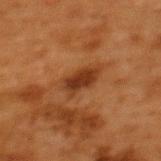On the upper back. The tile uses cross-polarized illumination. A roughly 15 mm field-of-view crop from a total-body skin photograph. Approximately 4 mm at its widest. A female subject approximately 50 years of age.